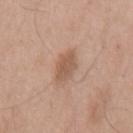Clinical impression: Part of a total-body skin-imaging series; this lesion was reviewed on a skin check and was not flagged for biopsy. Context: Automated tile analysis of the lesion measured a lesion area of about 5 mm², an eccentricity of roughly 0.75, and two-axis asymmetry of about 0.15. The analysis additionally found a classifier nevus-likeness of about 15/100 and a lesion-detection confidence of about 100/100. Located on the mid back. A 15 mm close-up tile from a total-body photography series done for melanoma screening. The recorded lesion diameter is about 3 mm. A male patient approximately 75 years of age.